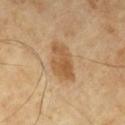This lesion was catalogued during total-body skin photography and was not selected for biopsy.
A 15 mm close-up extracted from a 3D total-body photography capture.
About 4.5 mm across.
The subject is a male in their mid-60s.
The lesion-visualizer software estimated a lesion area of about 11 mm² and a shape-asymmetry score of about 0.25 (0 = symmetric). It also reported roughly 9 lightness units darker than nearby skin and a normalized lesion–skin contrast near 7. The analysis additionally found a classifier nevus-likeness of about 85/100 and a lesion-detection confidence of about 100/100.
This is a cross-polarized tile.